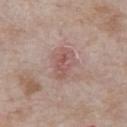The lesion was photographed on a routine skin check and not biopsied; there is no pathology result.
A 15 mm close-up tile from a total-body photography series done for melanoma screening.
The subject is a male in their mid- to late 60s.
The lesion is on the chest.
The total-body-photography lesion software estimated a mean CIELAB color near L≈55 a*≈21 b*≈22, about 8 CIELAB-L* units darker than the surrounding skin, and a normalized border contrast of about 6. It also reported a lesion-detection confidence of about 100/100.